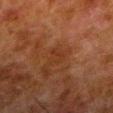Impression:
The lesion was photographed on a routine skin check and not biopsied; there is no pathology result.
Clinical summary:
The recorded lesion diameter is about 2.5 mm. Automated tile analysis of the lesion measured a nevus-likeness score of about 0/100 and a lesion-detection confidence of about 100/100. Cropped from a total-body skin-imaging series; the visible field is about 15 mm. The tile uses cross-polarized illumination. On the left lower leg. The patient is a male roughly 80 years of age.